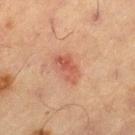The lesion was tiled from a total-body skin photograph and was not biopsied.
Imaged with cross-polarized lighting.
A male patient, aged 63 to 67.
A 15 mm close-up tile from a total-body photography series done for melanoma screening.
Located on the right thigh.
Longest diameter approximately 4 mm.